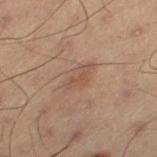Q: Was this lesion biopsied?
A: imaged on a skin check; not biopsied
Q: What lighting was used for the tile?
A: cross-polarized
Q: Automated lesion metrics?
A: a lesion area of about 6 mm² and an outline eccentricity of about 0.85 (0 = round, 1 = elongated); border irregularity of about 2.5 on a 0–10 scale, a color-variation rating of about 2.5/10, and peripheral color asymmetry of about 1; an automated nevus-likeness rating near 5 out of 100 and a lesion-detection confidence of about 100/100
Q: Lesion location?
A: the left thigh
Q: Patient demographics?
A: male, in their mid-50s
Q: How large is the lesion?
A: about 4 mm
Q: What is the imaging modality?
A: ~15 mm tile from a whole-body skin photo